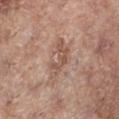Impression:
Captured during whole-body skin photography for melanoma surveillance; the lesion was not biopsied.
Context:
About 5 mm across. A female subject, in their mid-70s. Cropped from a whole-body photographic skin survey; the tile spans about 15 mm. Located on the left lower leg. Imaged with white-light lighting.